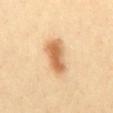| feature | finding |
|---|---|
| follow-up | no biopsy performed (imaged during a skin exam) |
| image | ~15 mm crop, total-body skin-cancer survey |
| diameter | ≈4.5 mm |
| lighting | cross-polarized |
| patient | female, roughly 40 years of age |
| site | the mid back |